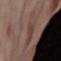Q: Was this lesion biopsied?
A: no biopsy performed (imaged during a skin exam)
Q: What is the anatomic site?
A: the abdomen
Q: Automated lesion metrics?
A: an outline eccentricity of about 0.95 (0 = round, 1 = elongated); an average lesion color of about L≈41 a*≈16 b*≈25 (CIELAB), about 6 CIELAB-L* units darker than the surrounding skin, and a normalized border contrast of about 5.5; a border-irregularity rating of about 3.5/10, a within-lesion color-variation index near 1/10, and radial color variation of about 0
Q: Patient demographics?
A: female, aged approximately 55
Q: How large is the lesion?
A: about 4.5 mm
Q: How was the tile lit?
A: cross-polarized
Q: What is the imaging modality?
A: ~15 mm tile from a whole-body skin photo